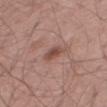<record>
  <biopsy_status>not biopsied; imaged during a skin examination</biopsy_status>
  <site>leg</site>
  <lighting>white-light</lighting>
  <patient>
    <sex>male</sex>
    <age_approx>70</age_approx>
  </patient>
  <lesion_size>
    <long_diameter_mm_approx>3.0</long_diameter_mm_approx>
  </lesion_size>
  <automated_metrics>
    <area_mm2_approx>4.0</area_mm2_approx>
    <shape_asymmetry>0.3</shape_asymmetry>
    <vs_skin_darker_L>10.0</vs_skin_darker_L>
    <vs_skin_contrast_norm>7.5</vs_skin_contrast_norm>
    <color_variation_0_10>2.5</color_variation_0_10>
    <nevus_likeness_0_100>0</nevus_likeness_0_100>
    <lesion_detection_confidence_0_100>100</lesion_detection_confidence_0_100>
  </automated_metrics>
  <image>
    <source>total-body photography crop</source>
    <field_of_view_mm>15</field_of_view_mm>
  </image>
</record>